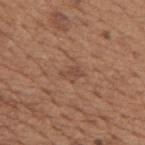<tbp_lesion>
<biopsy_status>not biopsied; imaged during a skin examination</biopsy_status>
<lesion_size>
  <long_diameter_mm_approx>2.5</long_diameter_mm_approx>
</lesion_size>
<automated_metrics>
  <cielab_L>46</cielab_L>
  <cielab_a>20</cielab_a>
  <cielab_b>28</cielab_b>
  <vs_skin_darker_L>7.0</vs_skin_darker_L>
  <vs_skin_contrast_norm>5.5</vs_skin_contrast_norm>
</automated_metrics>
<patient>
  <sex>male</sex>
  <age_approx>65</age_approx>
</patient>
<lighting>white-light</lighting>
<image>
  <source>total-body photography crop</source>
  <field_of_view_mm>15</field_of_view_mm>
</image>
<site>back</site>
</tbp_lesion>